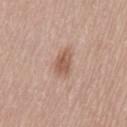The lesion was photographed on a routine skin check and not biopsied; there is no pathology result.
The recorded lesion diameter is about 3.5 mm.
A roughly 15 mm field-of-view crop from a total-body skin photograph.
From the lower back.
A male patient, approximately 75 years of age.
The total-body-photography lesion software estimated a lesion area of about 6.5 mm².
This is a white-light tile.